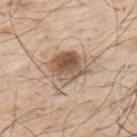Q: Was a biopsy performed?
A: imaged on a skin check; not biopsied
Q: Where on the body is the lesion?
A: the upper back
Q: What are the patient's age and sex?
A: male, about 70 years old
Q: What did automated image analysis measure?
A: a lesion area of about 16 mm² and an outline eccentricity of about 0.75 (0 = round, 1 = elongated); a lesion color around L≈57 a*≈16 b*≈29 in CIELAB, roughly 13 lightness units darker than nearby skin, and a normalized border contrast of about 8.5; a border-irregularity index near 4.5/10, internal color variation of about 8.5 on a 0–10 scale, and a peripheral color-asymmetry measure near 3; an automated nevus-likeness rating near 80 out of 100 and lesion-presence confidence of about 100/100
Q: What is the imaging modality?
A: ~15 mm crop, total-body skin-cancer survey
Q: How large is the lesion?
A: ≈6 mm